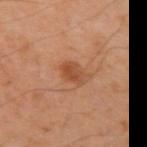Q: Was this lesion biopsied?
A: catalogued during a skin exam; not biopsied
Q: Illumination type?
A: cross-polarized
Q: What is the anatomic site?
A: the left upper arm
Q: What are the patient's age and sex?
A: male, aged 43–47
Q: Lesion size?
A: about 3 mm
Q: What kind of image is this?
A: total-body-photography crop, ~15 mm field of view
Q: Automated lesion metrics?
A: a lesion color around L≈48 a*≈25 b*≈35 in CIELAB, a lesion–skin lightness drop of about 9, and a normalized border contrast of about 7; border irregularity of about 2 on a 0–10 scale